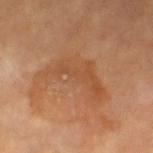biopsy status: catalogued during a skin exam; not biopsied | patient: female, about 70 years old | lighting: cross-polarized | TBP lesion metrics: a footprint of about 18 mm² and a shape-asymmetry score of about 0.6 (0 = symmetric); about 6 CIELAB-L* units darker than the surrounding skin and a normalized lesion–skin contrast near 4.5; an automated nevus-likeness rating near 0 out of 100 | lesion diameter: about 7.5 mm | image source: ~15 mm tile from a whole-body skin photo | location: the left thigh.